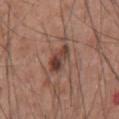Q: Was a biopsy performed?
A: catalogued during a skin exam; not biopsied
Q: What are the patient's age and sex?
A: male, aged around 55
Q: Automated lesion metrics?
A: a footprint of about 6.5 mm² and an outline eccentricity of about 0.85 (0 = round, 1 = elongated); a lesion–skin lightness drop of about 11 and a lesion-to-skin contrast of about 9 (normalized; higher = more distinct); a nevus-likeness score of about 55/100
Q: Lesion location?
A: the front of the torso
Q: What kind of image is this?
A: ~15 mm tile from a whole-body skin photo